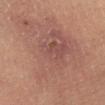* patient: male, aged around 60
* illumination: cross-polarized
* lesion diameter: ≈14.5 mm
* acquisition: ~15 mm tile from a whole-body skin photo